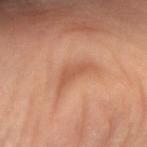Assessment: Imaged during a routine full-body skin examination; the lesion was not biopsied and no histopathology is available. Background: A female subject, aged 48–52. Approximately 5 mm at its widest. An algorithmic analysis of the crop reported a classifier nevus-likeness of about 0/100 and a detector confidence of about 75 out of 100 that the crop contains a lesion. The tile uses cross-polarized illumination. A 15 mm close-up tile from a total-body photography series done for melanoma screening. The lesion is located on the right forearm.